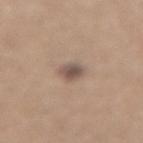Impression: No biopsy was performed on this lesion — it was imaged during a full skin examination and was not determined to be concerning. Acquisition and patient details: A roughly 15 mm field-of-view crop from a total-body skin photograph. Located on the lower back. Imaged with white-light lighting. The subject is a male aged 63 to 67. An algorithmic analysis of the crop reported a lesion area of about 5 mm², an outline eccentricity of about 0.7 (0 = round, 1 = elongated), and a shape-asymmetry score of about 0.2 (0 = symmetric). The analysis additionally found a border-irregularity rating of about 2/10 and a color-variation rating of about 3.5/10. The analysis additionally found an automated nevus-likeness rating near 70 out of 100 and lesion-presence confidence of about 100/100.